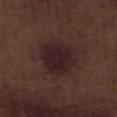The lesion was tiled from a total-body skin photograph and was not biopsied.
Approximately 5 mm at its widest.
The tile uses white-light illumination.
The lesion is on the right lower leg.
A region of skin cropped from a whole-body photographic capture, roughly 15 mm wide.
The patient is a male aged approximately 70.